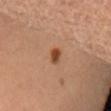Impression:
The lesion was photographed on a routine skin check and not biopsied; there is no pathology result.
Image and clinical context:
The recorded lesion diameter is about 2 mm. The patient is female. A close-up tile cropped from a whole-body skin photograph, about 15 mm across. The lesion is located on the back.